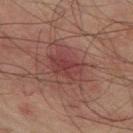The lesion is located on the leg. This image is a 15 mm lesion crop taken from a total-body photograph. Captured under cross-polarized illumination. A male patient, aged approximately 75. Measured at roughly 4.5 mm in maximum diameter.